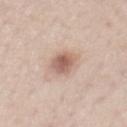Q: Is there a histopathology result?
A: catalogued during a skin exam; not biopsied
Q: Illumination type?
A: white-light
Q: What is the anatomic site?
A: the abdomen
Q: What kind of image is this?
A: 15 mm crop, total-body photography
Q: Patient demographics?
A: male, aged approximately 50